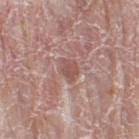Q: Was a biopsy performed?
A: total-body-photography surveillance lesion; no biopsy
Q: How large is the lesion?
A: ~2.5 mm (longest diameter)
Q: Patient demographics?
A: female, approximately 60 years of age
Q: What is the anatomic site?
A: the left thigh
Q: Illumination type?
A: white-light
Q: How was this image acquired?
A: ~15 mm tile from a whole-body skin photo